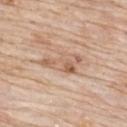workup: imaged on a skin check; not biopsied | anatomic site: the upper back | subject: female, in their 70s | image source: total-body-photography crop, ~15 mm field of view | automated metrics: a footprint of about 9.5 mm², a shape eccentricity near 0.9, and two-axis asymmetry of about 0.5; about 9 CIELAB-L* units darker than the surrounding skin | lesion size: about 5.5 mm.